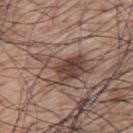Assessment: No biopsy was performed on this lesion — it was imaged during a full skin examination and was not determined to be concerning. Clinical summary: From the upper back. The patient is a male approximately 70 years of age. A 15 mm close-up extracted from a 3D total-body photography capture.